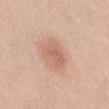This lesion was catalogued during total-body skin photography and was not selected for biopsy. This is a white-light tile. A female patient aged 28 to 32. The lesion is located on the right thigh. A 15 mm close-up extracted from a 3D total-body photography capture. About 3.5 mm across.